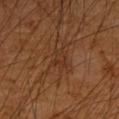workup: no biopsy performed (imaged during a skin exam)
lighting: cross-polarized illumination
patient: male, roughly 60 years of age
anatomic site: the arm
size: ≈3 mm
acquisition: 15 mm crop, total-body photography